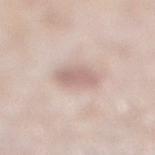Q: Is there a histopathology result?
A: catalogued during a skin exam; not biopsied
Q: Where on the body is the lesion?
A: the right lower leg
Q: How was this image acquired?
A: total-body-photography crop, ~15 mm field of view
Q: How was the tile lit?
A: white-light
Q: What are the patient's age and sex?
A: female, aged approximately 60
Q: Lesion size?
A: ≈3.5 mm